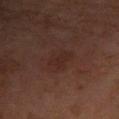Assessment:
No biopsy was performed on this lesion — it was imaged during a full skin examination and was not determined to be concerning.
Clinical summary:
A lesion tile, about 15 mm wide, cut from a 3D total-body photograph. Imaged with cross-polarized lighting. The lesion is on the right forearm. A female patient aged approximately 60. Measured at roughly 2.5 mm in maximum diameter.